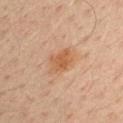| feature | finding |
|---|---|
| follow-up | catalogued during a skin exam; not biopsied |
| image source | ~15 mm tile from a whole-body skin photo |
| body site | the right upper arm |
| patient | male, in their mid- to late 30s |